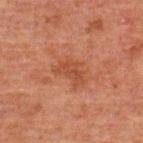The lesion was photographed on a routine skin check and not biopsied; there is no pathology result.
Automated tile analysis of the lesion measured a classifier nevus-likeness of about 0/100 and a detector confidence of about 100 out of 100 that the crop contains a lesion.
From the back.
The recorded lesion diameter is about 4 mm.
This is a cross-polarized tile.
The subject is a male approximately 60 years of age.
A region of skin cropped from a whole-body photographic capture, roughly 15 mm wide.